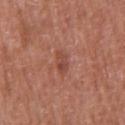Image and clinical context:
A region of skin cropped from a whole-body photographic capture, roughly 15 mm wide. The recorded lesion diameter is about 2.5 mm. Imaged with white-light lighting. On the chest. The patient is a male in their mid-60s.